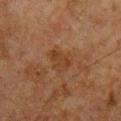<record>
<biopsy_status>not biopsied; imaged during a skin examination</biopsy_status>
<automated_metrics>
  <cielab_L>30</cielab_L>
  <cielab_a>17</cielab_a>
  <cielab_b>27</cielab_b>
  <vs_skin_contrast_norm>5.5</vs_skin_contrast_norm>
  <lesion_detection_confidence_0_100>100</lesion_detection_confidence_0_100>
</automated_metrics>
<lighting>cross-polarized</lighting>
<image>
  <source>total-body photography crop</source>
  <field_of_view_mm>15</field_of_view_mm>
</image>
<lesion_size>
  <long_diameter_mm_approx>3.5</long_diameter_mm_approx>
</lesion_size>
<site>chest</site>
<patient>
  <sex>male</sex>
  <age_approx>60</age_approx>
</patient>
</record>